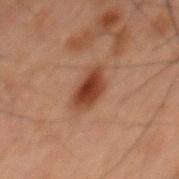Q: Is there a histopathology result?
A: no biopsy performed (imaged during a skin exam)
Q: How was the tile lit?
A: cross-polarized illumination
Q: What is the imaging modality?
A: ~15 mm crop, total-body skin-cancer survey
Q: How large is the lesion?
A: about 4.5 mm
Q: What is the anatomic site?
A: the mid back
Q: Patient demographics?
A: male, in their 60s